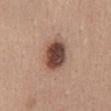Acquisition and patient details:
On the abdomen. A 15 mm close-up tile from a total-body photography series done for melanoma screening. A female patient, approximately 50 years of age.